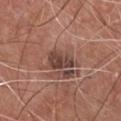Captured during whole-body skin photography for melanoma surveillance; the lesion was not biopsied. Captured under white-light illumination. From the chest. A roughly 15 mm field-of-view crop from a total-body skin photograph. The recorded lesion diameter is about 3.5 mm. Automated image analysis of the tile measured a lesion area of about 7 mm² and a symmetry-axis asymmetry near 0.3. The software also gave a lesion color around L≈42 a*≈19 b*≈23 in CIELAB and about 11 CIELAB-L* units darker than the surrounding skin. It also reported a border-irregularity index near 3.5/10, internal color variation of about 3 on a 0–10 scale, and peripheral color asymmetry of about 1. A male subject aged 73 to 77.